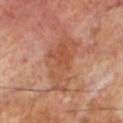{"biopsy_status": "not biopsied; imaged during a skin examination", "patient": {"sex": "male", "age_approx": 70}, "lesion_size": {"long_diameter_mm_approx": 7.5}, "site": "left lower leg", "image": {"source": "total-body photography crop", "field_of_view_mm": 15}}